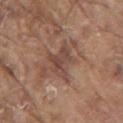Assessment: Recorded during total-body skin imaging; not selected for excision or biopsy. Image and clinical context: A 15 mm close-up extracted from a 3D total-body photography capture. The lesion's longest dimension is about 4 mm. Automated tile analysis of the lesion measured a shape eccentricity near 0.6 and a shape-asymmetry score of about 0.45 (0 = symmetric). And it measured a mean CIELAB color near L≈46 a*≈19 b*≈25, a lesion–skin lightness drop of about 8, and a normalized lesion–skin contrast near 6.5. A male subject, about 80 years old. The lesion is on the arm. The tile uses white-light illumination.